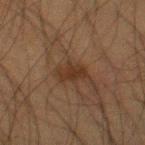workup — no biopsy performed (imaged during a skin exam)
imaging modality — ~15 mm crop, total-body skin-cancer survey
lesion diameter — ≈3.5 mm
location — the right thigh
illumination — cross-polarized illumination
TBP lesion metrics — a shape eccentricity near 0.8 and two-axis asymmetry of about 0.3; a border-irregularity rating of about 3.5/10, internal color variation of about 1.5 on a 0–10 scale, and radial color variation of about 0.5
patient — male, aged approximately 35